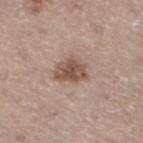  image:
    source: total-body photography crop
    field_of_view_mm: 15
  patient:
    sex: male
    age_approx: 70
  site: right leg